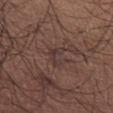biopsy_status: not biopsied; imaged during a skin examination
image:
  source: total-body photography crop
  field_of_view_mm: 15
lighting: white-light
patient:
  sex: male
  age_approx: 65
automated_metrics:
  area_mm2_approx: 4.0
  eccentricity: 0.75
  cielab_L: 35
  cielab_a: 16
  cielab_b: 19
  border_irregularity_0_10: 6.0
  color_variation_0_10: 1.0
  peripheral_color_asymmetry: 0.0
site: abdomen
lesion_size:
  long_diameter_mm_approx: 3.0